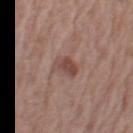No biopsy was performed on this lesion — it was imaged during a full skin examination and was not determined to be concerning.
Measured at roughly 3 mm in maximum diameter.
A 15 mm close-up tile from a total-body photography series done for melanoma screening.
The lesion is on the arm.
A male subject, aged around 70.
Imaged with white-light lighting.